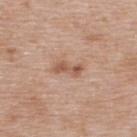Captured during whole-body skin photography for melanoma surveillance; the lesion was not biopsied.